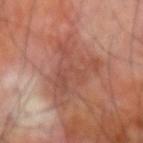Recorded during total-body skin imaging; not selected for excision or biopsy. Located on the right forearm. A roughly 15 mm field-of-view crop from a total-body skin photograph. Captured under cross-polarized illumination. A male patient roughly 70 years of age. Longest diameter approximately 6.5 mm.